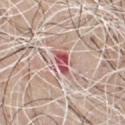Q: Was a biopsy performed?
A: catalogued during a skin exam; not biopsied
Q: What is the imaging modality?
A: 15 mm crop, total-body photography
Q: What are the patient's age and sex?
A: male, approximately 70 years of age
Q: What is the anatomic site?
A: the chest